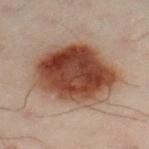<tbp_lesion>
  <biopsy_status>not biopsied; imaged during a skin examination</biopsy_status>
  <lighting>cross-polarized</lighting>
  <patient>
    <sex>male</sex>
    <age_approx>50</age_approx>
  </patient>
  <image>
    <source>total-body photography crop</source>
    <field_of_view_mm>15</field_of_view_mm>
  </image>
  <site>left leg</site>
</tbp_lesion>